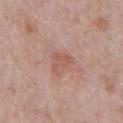  biopsy_status: not biopsied; imaged during a skin examination
  lighting: white-light
  patient:
    sex: male
    age_approx: 70
  automated_metrics:
    border_irregularity_0_10: 5.0
    color_variation_0_10: 1.0
    peripheral_color_asymmetry: 0.5
    nevus_likeness_0_100: 0
    lesion_detection_confidence_0_100: 100
  lesion_size:
    long_diameter_mm_approx: 3.0
  site: abdomen
  image:
    source: total-body photography crop
    field_of_view_mm: 15